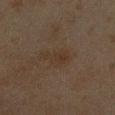Clinical impression:
Part of a total-body skin-imaging series; this lesion was reviewed on a skin check and was not flagged for biopsy.
Acquisition and patient details:
Cropped from a total-body skin-imaging series; the visible field is about 15 mm. About 4 mm across. The lesion is on the right forearm. A male patient roughly 45 years of age. Captured under cross-polarized illumination.